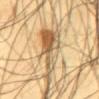* biopsy status · total-body-photography surveillance lesion; no biopsy
* size · ~7 mm (longest diameter)
* patient · male, about 45 years old
* site · the upper back
* image-analysis metrics · two-axis asymmetry of about 0.6; an average lesion color of about L≈59 a*≈15 b*≈37 (CIELAB), roughly 13 lightness units darker than nearby skin, and a normalized lesion–skin contrast near 8; border irregularity of about 7.5 on a 0–10 scale, a within-lesion color-variation index near 10/10, and a peripheral color-asymmetry measure near 3.5
* image source · ~15 mm tile from a whole-body skin photo